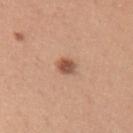{"biopsy_status": "not biopsied; imaged during a skin examination", "lesion_size": {"long_diameter_mm_approx": 2.5}, "automated_metrics": {"cielab_L": 54, "cielab_a": 22, "cielab_b": 31, "vs_skin_darker_L": 13.0, "vs_skin_contrast_norm": 9.0, "nevus_likeness_0_100": 95, "lesion_detection_confidence_0_100": 100}, "site": "right upper arm", "lighting": "white-light", "image": {"source": "total-body photography crop", "field_of_view_mm": 15}, "patient": {"sex": "female", "age_approx": 30}}